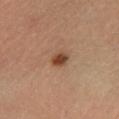Captured during whole-body skin photography for melanoma surveillance; the lesion was not biopsied.
The lesion is located on the left lower leg.
A region of skin cropped from a whole-body photographic capture, roughly 15 mm wide.
Captured under cross-polarized illumination.
A female patient in their mid- to late 30s.
The lesion-visualizer software estimated an area of roughly 3 mm², an outline eccentricity of about 0.7 (0 = round, 1 = elongated), and two-axis asymmetry of about 0.2. And it measured a mean CIELAB color near L≈42 a*≈22 b*≈32, roughly 13 lightness units darker than nearby skin, and a normalized border contrast of about 10.5.